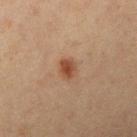Findings:
– follow-up — catalogued during a skin exam; not biopsied
– diameter — ≈2.5 mm
– patient — female, about 40 years old
– imaging modality — 15 mm crop, total-body photography
– anatomic site — the right upper arm